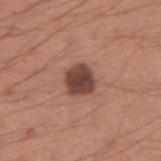biopsy status = imaged on a skin check; not biopsied | lesion diameter = about 3 mm | automated lesion analysis = border irregularity of about 1.5 on a 0–10 scale, a color-variation rating of about 3/10, and radial color variation of about 1; a nevus-likeness score of about 45/100 | subject = male, aged 33 to 37 | image source = ~15 mm crop, total-body skin-cancer survey | location = the right upper arm | illumination = white-light illumination.